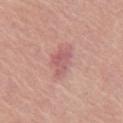Clinical impression: Part of a total-body skin-imaging series; this lesion was reviewed on a skin check and was not flagged for biopsy. Context: From the chest. Longest diameter approximately 3.5 mm. Captured under white-light illumination. The subject is a male roughly 60 years of age. A roughly 15 mm field-of-view crop from a total-body skin photograph.